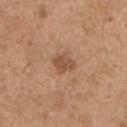The lesion was photographed on a routine skin check and not biopsied; there is no pathology result.
A male subject aged 63 to 67.
Measured at roughly 3 mm in maximum diameter.
A roughly 15 mm field-of-view crop from a total-body skin photograph.
Located on the chest.